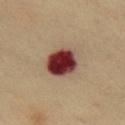Captured during whole-body skin photography for melanoma surveillance; the lesion was not biopsied.
Cropped from a whole-body photographic skin survey; the tile spans about 15 mm.
The total-body-photography lesion software estimated border irregularity of about 1 on a 0–10 scale, internal color variation of about 7 on a 0–10 scale, and peripheral color asymmetry of about 1.5. The analysis additionally found an automated nevus-likeness rating near 0 out of 100 and a detector confidence of about 100 out of 100 that the crop contains a lesion.
The recorded lesion diameter is about 4 mm.
On the chest.
This is a cross-polarized tile.
The subject is a female in their 60s.